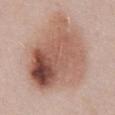Imaged during a routine full-body skin examination; the lesion was not biopsied and no histopathology is available. A male subject about 55 years old. This image is a 15 mm lesion crop taken from a total-body photograph. The lesion is on the abdomen.